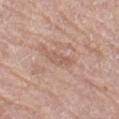Imaged during a routine full-body skin examination; the lesion was not biopsied and no histopathology is available. The patient is a female aged approximately 60. A lesion tile, about 15 mm wide, cut from a 3D total-body photograph. This is a white-light tile. On the left lower leg.